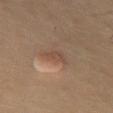A male patient in their mid-50s.
A region of skin cropped from a whole-body photographic capture, roughly 15 mm wide.
Captured under cross-polarized illumination.
The lesion is located on the chest.